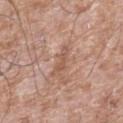Q: What lighting was used for the tile?
A: white-light illumination
Q: What are the patient's age and sex?
A: male, aged 58 to 62
Q: What is the imaging modality?
A: ~15 mm tile from a whole-body skin photo
Q: What is the anatomic site?
A: the right lower leg
Q: Automated lesion metrics?
A: a nevus-likeness score of about 0/100
Q: Lesion size?
A: ≈3.5 mm